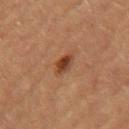No biopsy was performed on this lesion — it was imaged during a full skin examination and was not determined to be concerning.